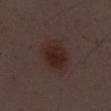Longest diameter approximately 4.5 mm. This image is a 15 mm lesion crop taken from a total-body photograph. A male patient, approximately 50 years of age. This is a white-light tile.